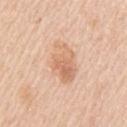{"biopsy_status": "not biopsied; imaged during a skin examination", "image": {"source": "total-body photography crop", "field_of_view_mm": 15}, "lighting": "white-light", "site": "left upper arm", "patient": {"sex": "female", "age_approx": 45}}